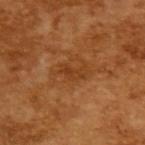Assessment:
Captured during whole-body skin photography for melanoma surveillance; the lesion was not biopsied.
Background:
Longest diameter approximately 3 mm. A male patient in their mid- to late 60s. Captured under cross-polarized illumination. An algorithmic analysis of the crop reported a lesion area of about 3.5 mm², an eccentricity of roughly 0.85, and a shape-asymmetry score of about 0.5 (0 = symmetric). The analysis additionally found a nevus-likeness score of about 0/100. A roughly 15 mm field-of-view crop from a total-body skin photograph.